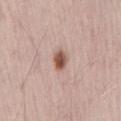Recorded during total-body skin imaging; not selected for excision or biopsy.
A 15 mm crop from a total-body photograph taken for skin-cancer surveillance.
Longest diameter approximately 2.5 mm.
Imaged with white-light lighting.
A male subject aged approximately 70.
The lesion is on the lower back.